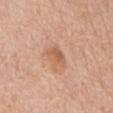Impression:
Captured during whole-body skin photography for melanoma surveillance; the lesion was not biopsied.
Context:
On the front of the torso. A 15 mm crop from a total-body photograph taken for skin-cancer surveillance. Automated image analysis of the tile measured a lesion area of about 4.5 mm² and a shape-asymmetry score of about 0.25 (0 = symmetric). A female patient, aged 73 to 77.